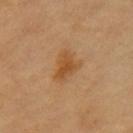Clinical impression: This lesion was catalogued during total-body skin photography and was not selected for biopsy. Context: The patient is female. Cropped from a total-body skin-imaging series; the visible field is about 15 mm. Captured under cross-polarized illumination.